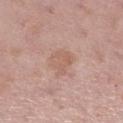This lesion was catalogued during total-body skin photography and was not selected for biopsy. The subject is a female approximately 50 years of age. The lesion's longest dimension is about 3.5 mm. A 15 mm close-up extracted from a 3D total-body photography capture. The lesion is located on the right lower leg. Captured under white-light illumination.